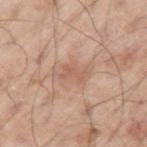About 3 mm across.
Automated image analysis of the tile measured an area of roughly 3.5 mm², an outline eccentricity of about 0.8 (0 = round, 1 = elongated), and a shape-asymmetry score of about 0.45 (0 = symmetric).
A roughly 15 mm field-of-view crop from a total-body skin photograph.
The lesion is on the left upper arm.
This is a white-light tile.
A male subject, aged approximately 70.